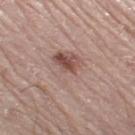The lesion was tiled from a total-body skin photograph and was not biopsied. This is a white-light tile. A 15 mm close-up extracted from a 3D total-body photography capture. Approximately 5.5 mm at its widest. A male subject, in their 70s. The lesion is located on the left leg.